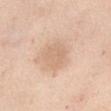The lesion was photographed on a routine skin check and not biopsied; there is no pathology result. The patient is a female aged 53 to 57. The lesion-visualizer software estimated a footprint of about 9 mm², a shape eccentricity near 0.2, and a shape-asymmetry score of about 0.2 (0 = symmetric). The lesion is on the abdomen. Captured under white-light illumination. A close-up tile cropped from a whole-body skin photograph, about 15 mm across. The recorded lesion diameter is about 3.5 mm.